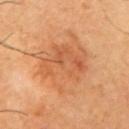Clinical impression:
The lesion was photographed on a routine skin check and not biopsied; there is no pathology result.
Clinical summary:
The subject is a male roughly 65 years of age. Longest diameter approximately 8.5 mm. Imaged with cross-polarized lighting. The lesion is on the arm. A roughly 15 mm field-of-view crop from a total-body skin photograph.